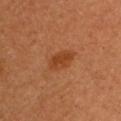Imaged during a routine full-body skin examination; the lesion was not biopsied and no histopathology is available.
A female subject approximately 50 years of age.
This is a cross-polarized tile.
The lesion-visualizer software estimated a classifier nevus-likeness of about 95/100 and a detector confidence of about 100 out of 100 that the crop contains a lesion.
A 15 mm crop from a total-body photograph taken for skin-cancer surveillance.
About 3.5 mm across.